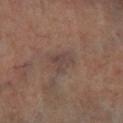The lesion was photographed on a routine skin check and not biopsied; there is no pathology result. The tile uses cross-polarized illumination. On the right lower leg. The recorded lesion diameter is about 3 mm. Automated image analysis of the tile measured a lesion–skin lightness drop of about 6 and a normalized border contrast of about 6. The analysis additionally found an automated nevus-likeness rating near 0 out of 100 and a lesion-detection confidence of about 80/100. This image is a 15 mm lesion crop taken from a total-body photograph. A male subject aged 63 to 67.